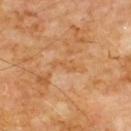Imaged during a routine full-body skin examination; the lesion was not biopsied and no histopathology is available. The patient is a male in their 60s. A 15 mm close-up extracted from a 3D total-body photography capture. Approximately 3 mm at its widest. An algorithmic analysis of the crop reported a lesion area of about 2.5 mm², an outline eccentricity of about 0.95 (0 = round, 1 = elongated), and a shape-asymmetry score of about 0.45 (0 = symmetric). The analysis additionally found a lesion color around L≈55 a*≈22 b*≈40 in CIELAB, a lesion–skin lightness drop of about 5, and a lesion-to-skin contrast of about 4.5 (normalized; higher = more distinct). And it measured a border-irregularity index near 7/10 and internal color variation of about 0 on a 0–10 scale. The lesion is located on the front of the torso. The tile uses cross-polarized illumination.